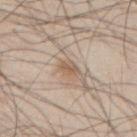anatomic site=the upper back; acquisition=15 mm crop, total-body photography; patient=male, about 45 years old.